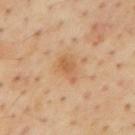The lesion was photographed on a routine skin check and not biopsied; there is no pathology result.
A lesion tile, about 15 mm wide, cut from a 3D total-body photograph.
A male patient aged approximately 55.
Imaged with cross-polarized lighting.
The lesion-visualizer software estimated an average lesion color of about L≈60 a*≈21 b*≈38 (CIELAB), about 8 CIELAB-L* units darker than the surrounding skin, and a normalized lesion–skin contrast near 6. It also reported a within-lesion color-variation index near 3/10 and radial color variation of about 1.
On the mid back.